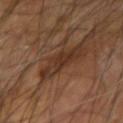On the left forearm.
This image is a 15 mm lesion crop taken from a total-body photograph.
A male subject, aged 63–67.